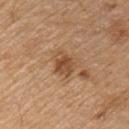The lesion was photographed on a routine skin check and not biopsied; there is no pathology result.
The patient is a male in their 70s.
The tile uses white-light illumination.
Automated image analysis of the tile measured a lesion color around L≈49 a*≈21 b*≈34 in CIELAB and a lesion-to-skin contrast of about 8 (normalized; higher = more distinct). The analysis additionally found border irregularity of about 2.5 on a 0–10 scale. It also reported a nevus-likeness score of about 60/100 and a lesion-detection confidence of about 100/100.
Cropped from a total-body skin-imaging series; the visible field is about 15 mm.
The lesion is located on the chest.